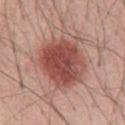- biopsy status — total-body-photography surveillance lesion; no biopsy
- site — the chest
- size — ≈6 mm
- image — ~15 mm crop, total-body skin-cancer survey
- subject — male, roughly 65 years of age